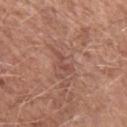biopsy status: no biopsy performed (imaged during a skin exam) | image source: ~15 mm crop, total-body skin-cancer survey | lighting: white-light | image-analysis metrics: a lesion area of about 3 mm², an eccentricity of roughly 0.95, and a shape-asymmetry score of about 0.55 (0 = symmetric); a lesion color around L≈49 a*≈23 b*≈26 in CIELAB and a lesion–skin lightness drop of about 7; a color-variation rating of about 0/10 and peripheral color asymmetry of about 0; a lesion-detection confidence of about 70/100 | patient: male, approximately 50 years of age | diameter: ≈3 mm | anatomic site: the left upper arm.